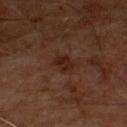The lesion was tiled from a total-body skin photograph and was not biopsied.
A male patient, roughly 55 years of age.
The lesion is located on the front of the torso.
The lesion's longest dimension is about 2.5 mm.
Imaged with cross-polarized lighting.
A roughly 15 mm field-of-view crop from a total-body skin photograph.
The lesion-visualizer software estimated an automated nevus-likeness rating near 30 out of 100.